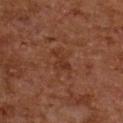Acquisition and patient details: The tile uses cross-polarized illumination. Automated tile analysis of the lesion measured a footprint of about 3 mm² and two-axis asymmetry of about 0.5. The analysis additionally found roughly 5 lightness units darker than nearby skin and a lesion-to-skin contrast of about 5.5 (normalized; higher = more distinct). Measured at roughly 2.5 mm in maximum diameter. The patient is a female roughly 60 years of age. The lesion is on the upper back. A close-up tile cropped from a whole-body skin photograph, about 15 mm across.